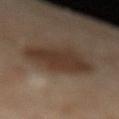biopsy status: catalogued during a skin exam; not biopsied
size: ~5 mm (longest diameter)
automated metrics: a footprint of about 19 mm², an eccentricity of roughly 0.5, and two-axis asymmetry of about 0.15; a lesion color around L≈33 a*≈15 b*≈24 in CIELAB and a lesion-to-skin contrast of about 8.5 (normalized; higher = more distinct); a classifier nevus-likeness of about 95/100 and a lesion-detection confidence of about 100/100
illumination: cross-polarized
image source: ~15 mm tile from a whole-body skin photo
body site: the left lower leg
patient: female, in their 60s